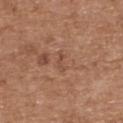Clinical impression: Imaged during a routine full-body skin examination; the lesion was not biopsied and no histopathology is available.